A female subject, aged approximately 40; Automated image analysis of the tile measured a footprint of about 34 mm², an eccentricity of roughly 0.65, and two-axis asymmetry of about 0.1. The software also gave a border-irregularity rating of about 1.5/10 and radial color variation of about 2.5. And it measured an automated nevus-likeness rating near 100 out of 100; measured at roughly 7.5 mm in maximum diameter; a region of skin cropped from a whole-body photographic capture, roughly 15 mm wide; the lesion is located on the mid back; imaged with cross-polarized lighting — 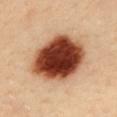biopsy diagnosis = a compound melanocytic nevus (benign).